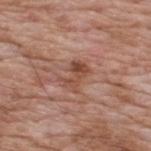Imaged with white-light lighting. A male patient, aged 58–62. A close-up tile cropped from a whole-body skin photograph, about 15 mm across. About 3 mm across. Located on the upper back.